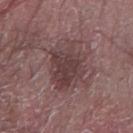Recorded during total-body skin imaging; not selected for excision or biopsy. An algorithmic analysis of the crop reported a lesion color around L≈40 a*≈18 b*≈16 in CIELAB, about 9 CIELAB-L* units darker than the surrounding skin, and a lesion-to-skin contrast of about 7.5 (normalized; higher = more distinct). Located on the right forearm. This is a white-light tile. A male patient in their mid-60s. A close-up tile cropped from a whole-body skin photograph, about 15 mm across. Longest diameter approximately 6 mm.